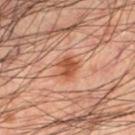Findings:
• workup: catalogued during a skin exam; not biopsied
• automated metrics: an area of roughly 5.5 mm² and a shape-asymmetry score of about 0.25 (0 = symmetric); an average lesion color of about L≈39 a*≈21 b*≈28 (CIELAB), roughly 9 lightness units darker than nearby skin, and a normalized lesion–skin contrast near 8; a classifier nevus-likeness of about 90/100 and lesion-presence confidence of about 100/100
• lesion diameter: ≈2.5 mm
• site: the left lower leg
• patient: male, aged around 50
• acquisition: ~15 mm tile from a whole-body skin photo
• illumination: cross-polarized illumination